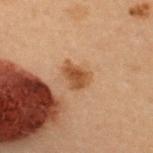Clinical impression: The lesion was photographed on a routine skin check and not biopsied; there is no pathology result. Clinical summary: Automated tile analysis of the lesion measured an area of roughly 6 mm² and a shape-asymmetry score of about 0.3 (0 = symmetric). The analysis additionally found an average lesion color of about L≈40 a*≈19 b*≈31 (CIELAB), about 10 CIELAB-L* units darker than the surrounding skin, and a normalized border contrast of about 8.5. The analysis additionally found a border-irregularity index near 2.5/10 and a within-lesion color-variation index near 2.5/10. Located on the upper back. This image is a 15 mm lesion crop taken from a total-body photograph. The tile uses cross-polarized illumination. The lesion's longest dimension is about 3 mm. The subject is a female aged 28 to 32.